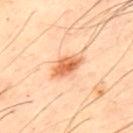| key | value |
|---|---|
| notes | total-body-photography surveillance lesion; no biopsy |
| body site | the upper back |
| subject | male, in their 50s |
| size | ~4 mm (longest diameter) |
| TBP lesion metrics | an average lesion color of about L≈67 a*≈28 b*≈41 (CIELAB), about 15 CIELAB-L* units darker than the surrounding skin, and a lesion-to-skin contrast of about 9.5 (normalized; higher = more distinct) |
| image | ~15 mm crop, total-body skin-cancer survey |
| illumination | cross-polarized |